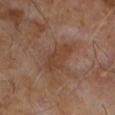Clinical impression: This lesion was catalogued during total-body skin photography and was not selected for biopsy. Image and clinical context: Automated image analysis of the tile measured a nevus-likeness score of about 0/100 and a detector confidence of about 100 out of 100 that the crop contains a lesion. This is a cross-polarized tile. A male patient, aged around 65. A region of skin cropped from a whole-body photographic capture, roughly 15 mm wide.